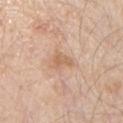Clinical impression:
This lesion was catalogued during total-body skin photography and was not selected for biopsy.
Context:
A male subject approximately 80 years of age. Longest diameter approximately 3 mm. The lesion-visualizer software estimated an area of roughly 4 mm², an outline eccentricity of about 0.75 (0 = round, 1 = elongated), and two-axis asymmetry of about 0.45. And it measured border irregularity of about 4.5 on a 0–10 scale, internal color variation of about 1.5 on a 0–10 scale, and peripheral color asymmetry of about 0.5. The lesion is located on the chest. A 15 mm crop from a total-body photograph taken for skin-cancer surveillance.